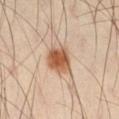notes — no biopsy performed (imaged during a skin exam) | size — about 3.5 mm | image source — ~15 mm tile from a whole-body skin photo | TBP lesion metrics — an average lesion color of about L≈55 a*≈21 b*≈34 (CIELAB), a lesion–skin lightness drop of about 15, and a normalized lesion–skin contrast near 10.5; peripheral color asymmetry of about 1.5; a detector confidence of about 100 out of 100 that the crop contains a lesion | body site — the leg | subject — male, aged 38–42 | tile lighting — cross-polarized.